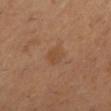Captured during whole-body skin photography for melanoma surveillance; the lesion was not biopsied. Automated image analysis of the tile measured a border-irregularity index near 2/10 and a color-variation rating of about 1.5/10. It also reported a nevus-likeness score of about 10/100 and a detector confidence of about 100 out of 100 that the crop contains a lesion. The subject is a female approximately 55 years of age. Cropped from a whole-body photographic skin survey; the tile spans about 15 mm. Captured under cross-polarized illumination. The lesion is located on the left lower leg.